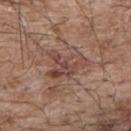This lesion was catalogued during total-body skin photography and was not selected for biopsy.
A male subject in their mid-60s.
The lesion is located on the back.
Captured under white-light illumination.
Cropped from a whole-body photographic skin survey; the tile spans about 15 mm.
The lesion's longest dimension is about 5 mm.